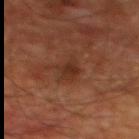Q: Was a biopsy performed?
A: imaged on a skin check; not biopsied
Q: What did automated image analysis measure?
A: a classifier nevus-likeness of about 0/100 and a detector confidence of about 100 out of 100 that the crop contains a lesion
Q: What kind of image is this?
A: ~15 mm tile from a whole-body skin photo
Q: What are the patient's age and sex?
A: male, about 60 years old
Q: What is the anatomic site?
A: the chest